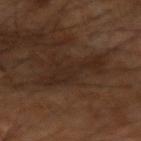Assessment: The lesion was photographed on a routine skin check and not biopsied; there is no pathology result. Acquisition and patient details: The tile uses cross-polarized illumination. Longest diameter approximately 5.5 mm. Located on the left arm. The subject is a male roughly 60 years of age. A close-up tile cropped from a whole-body skin photograph, about 15 mm across.